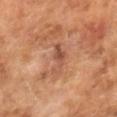  biopsy_status: not biopsied; imaged during a skin examination
  patient:
    sex: male
    age_approx: 65
  lighting: cross-polarized
  image:
    source: total-body photography crop
    field_of_view_mm: 15
  lesion_size:
    long_diameter_mm_approx: 4.0